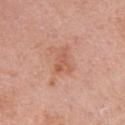• size: ~3 mm (longest diameter)
• site: the left upper arm
• patient: female, aged approximately 55
• image source: total-body-photography crop, ~15 mm field of view
• illumination: white-light